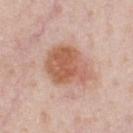biopsy status = imaged on a skin check; not biopsied | image source = ~15 mm crop, total-body skin-cancer survey | body site = the chest | lighting = white-light | patient = male, in their 60s | lesion diameter = ≈5.5 mm.